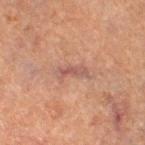Q: Is there a histopathology result?
A: no biopsy performed (imaged during a skin exam)
Q: Where on the body is the lesion?
A: the right thigh
Q: Patient demographics?
A: female, aged approximately 60
Q: Lesion size?
A: about 3.5 mm
Q: What kind of image is this?
A: ~15 mm tile from a whole-body skin photo
Q: How was the tile lit?
A: cross-polarized